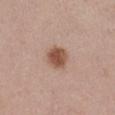follow-up=no biopsy performed (imaged during a skin exam) | image source=~15 mm crop, total-body skin-cancer survey | subject=female, roughly 40 years of age | body site=the left thigh | diameter=about 3 mm | lighting=white-light.